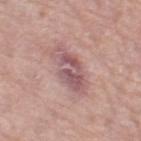No biopsy was performed on this lesion — it was imaged during a full skin examination and was not determined to be concerning. A 15 mm close-up extracted from a 3D total-body photography capture. Located on the left thigh. The tile uses white-light illumination. The subject is a female aged 63 to 67. The recorded lesion diameter is about 6 mm.